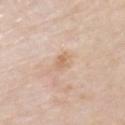The lesion was tiled from a total-body skin photograph and was not biopsied.
From the left upper arm.
Cropped from a total-body skin-imaging series; the visible field is about 15 mm.
Captured under white-light illumination.
The subject is a male roughly 75 years of age.
Automated image analysis of the tile measured a footprint of about 3.5 mm² and an eccentricity of roughly 0.9. And it measured a lesion color around L≈66 a*≈18 b*≈32 in CIELAB, about 8 CIELAB-L* units darker than the surrounding skin, and a lesion-to-skin contrast of about 6 (normalized; higher = more distinct). The analysis additionally found a border-irregularity rating of about 4.5/10, a color-variation rating of about 1/10, and a peripheral color-asymmetry measure near 0.5.